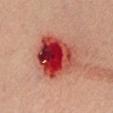Assessment: This lesion was catalogued during total-body skin photography and was not selected for biopsy. Context: A male patient approximately 35 years of age. The tile uses white-light illumination. The lesion-visualizer software estimated a nevus-likeness score of about 0/100 and lesion-presence confidence of about 100/100. From the left lower leg. About 7 mm across. This image is a 15 mm lesion crop taken from a total-body photograph.